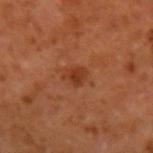Clinical impression: Imaged during a routine full-body skin examination; the lesion was not biopsied and no histopathology is available. Image and clinical context: On the left forearm. The lesion-visualizer software estimated an area of roughly 4 mm², an outline eccentricity of about 0.55 (0 = round, 1 = elongated), and a symmetry-axis asymmetry near 0.3. The analysis additionally found an average lesion color of about L≈35 a*≈26 b*≈33 (CIELAB) and about 8 CIELAB-L* units darker than the surrounding skin. And it measured border irregularity of about 3 on a 0–10 scale, a within-lesion color-variation index near 2/10, and peripheral color asymmetry of about 0.5. The software also gave a classifier nevus-likeness of about 60/100 and a lesion-detection confidence of about 100/100. Approximately 2.5 mm at its widest. A male patient, roughly 60 years of age. A close-up tile cropped from a whole-body skin photograph, about 15 mm across. This is a cross-polarized tile.